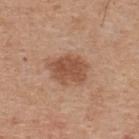follow-up = total-body-photography surveillance lesion; no biopsy